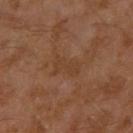Background:
A 15 mm close-up tile from a total-body photography series done for melanoma screening. The tile uses cross-polarized illumination. Measured at roughly 3.5 mm in maximum diameter. A male subject, aged approximately 30. Located on the left arm.